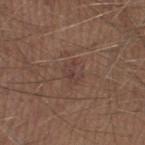This lesion was catalogued during total-body skin photography and was not selected for biopsy. A 15 mm crop from a total-body photograph taken for skin-cancer surveillance. A male subject, aged 28–32. Measured at roughly 2.5 mm in maximum diameter. From the left thigh. The total-body-photography lesion software estimated an area of roughly 3.5 mm², a shape eccentricity near 0.7, and a symmetry-axis asymmetry near 0.45. And it measured a border-irregularity index near 5/10, internal color variation of about 0.5 on a 0–10 scale, and radial color variation of about 0.